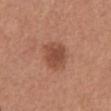The lesion was photographed on a routine skin check and not biopsied; there is no pathology result.
Cropped from a total-body skin-imaging series; the visible field is about 15 mm.
A male subject, about 65 years old.
On the abdomen.